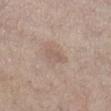Assessment:
Recorded during total-body skin imaging; not selected for excision or biopsy.
Clinical summary:
A female subject, in their mid-60s. Located on the right lower leg. A lesion tile, about 15 mm wide, cut from a 3D total-body photograph.